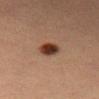Part of a total-body skin-imaging series; this lesion was reviewed on a skin check and was not flagged for biopsy.
Cropped from a whole-body photographic skin survey; the tile spans about 15 mm.
A female subject, aged 38–42.
This is a cross-polarized tile.
The lesion's longest dimension is about 3 mm.
On the leg.